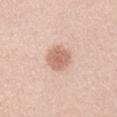Impression: This lesion was catalogued during total-body skin photography and was not selected for biopsy. Context: A male patient about 45 years old. From the right upper arm. Longest diameter approximately 3.5 mm. A 15 mm close-up extracted from a 3D total-body photography capture. This is a white-light tile.